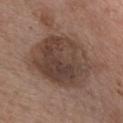  site: front of the torso
  image:
    source: total-body photography crop
    field_of_view_mm: 15
  patient:
    sex: male
    age_approx: 65